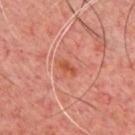Acquisition and patient details: A close-up tile cropped from a whole-body skin photograph, about 15 mm across. A male subject aged 43–47. Captured under cross-polarized illumination. On the chest.